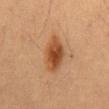A close-up tile cropped from a whole-body skin photograph, about 15 mm across. An algorithmic analysis of the crop reported a lesion color around L≈42 a*≈21 b*≈33 in CIELAB and about 11 CIELAB-L* units darker than the surrounding skin. Approximately 5 mm at its widest. The lesion is on the mid back. Captured under cross-polarized illumination. The subject is a male in their mid-50s.